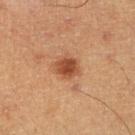Q: Is there a histopathology result?
A: total-body-photography surveillance lesion; no biopsy
Q: What kind of image is this?
A: ~15 mm tile from a whole-body skin photo
Q: What is the anatomic site?
A: the right lower leg
Q: Patient demographics?
A: male, aged 58–62